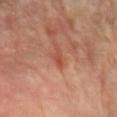Q: Is there a histopathology result?
A: imaged on a skin check; not biopsied
Q: Illumination type?
A: cross-polarized illumination
Q: Where on the body is the lesion?
A: the left forearm
Q: What are the patient's age and sex?
A: female, aged approximately 60
Q: What is the imaging modality?
A: total-body-photography crop, ~15 mm field of view
Q: Lesion size?
A: ≈2.5 mm